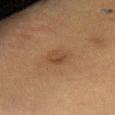The lesion was photographed on a routine skin check and not biopsied; there is no pathology result. Located on the right thigh. This is a cross-polarized tile. The patient is a female about 55 years old. A region of skin cropped from a whole-body photographic capture, roughly 15 mm wide. The lesion-visualizer software estimated a mean CIELAB color near L≈38 a*≈16 b*≈28, about 6 CIELAB-L* units darker than the surrounding skin, and a normalized border contrast of about 5.5. The software also gave internal color variation of about 4 on a 0–10 scale and peripheral color asymmetry of about 1.5. The analysis additionally found an automated nevus-likeness rating near 25 out of 100 and a detector confidence of about 100 out of 100 that the crop contains a lesion.